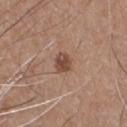This lesion was catalogued during total-body skin photography and was not selected for biopsy.
Imaged with white-light lighting.
Located on the chest.
About 2.5 mm across.
A male patient, aged around 55.
A roughly 15 mm field-of-view crop from a total-body skin photograph.
Automated tile analysis of the lesion measured a lesion–skin lightness drop of about 11 and a lesion-to-skin contrast of about 8.5 (normalized; higher = more distinct). It also reported border irregularity of about 2 on a 0–10 scale. It also reported an automated nevus-likeness rating near 85 out of 100 and a lesion-detection confidence of about 100/100.